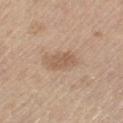No biopsy was performed on this lesion — it was imaged during a full skin examination and was not determined to be concerning.
The patient is a male aged 68 to 72.
Approximately 3.5 mm at its widest.
A 15 mm close-up extracted from a 3D total-body photography capture.
An algorithmic analysis of the crop reported a footprint of about 6 mm², an outline eccentricity of about 0.8 (0 = round, 1 = elongated), and a symmetry-axis asymmetry near 0.2. The software also gave roughly 8 lightness units darker than nearby skin and a lesion-to-skin contrast of about 6 (normalized; higher = more distinct). The analysis additionally found border irregularity of about 2 on a 0–10 scale, a within-lesion color-variation index near 2/10, and peripheral color asymmetry of about 0.5.
On the right thigh.
Captured under white-light illumination.